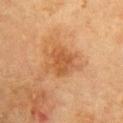patient = female, aged 78 to 82 | image = total-body-photography crop, ~15 mm field of view | body site = the right upper arm.